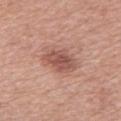Recorded during total-body skin imaging; not selected for excision or biopsy. Automated image analysis of the tile measured a border-irregularity index near 2.5/10, a color-variation rating of about 3.5/10, and a peripheral color-asymmetry measure near 1. The software also gave a classifier nevus-likeness of about 50/100 and a detector confidence of about 100 out of 100 that the crop contains a lesion. The lesion is located on the mid back. Longest diameter approximately 4 mm. The subject is a male about 65 years old. A region of skin cropped from a whole-body photographic capture, roughly 15 mm wide.